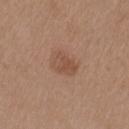{"biopsy_status": "not biopsied; imaged during a skin examination", "site": "right upper arm", "lighting": "white-light", "automated_metrics": {"border_irregularity_0_10": 2.0, "color_variation_0_10": 2.5, "peripheral_color_asymmetry": 1.0}, "patient": {"sex": "female", "age_approx": 40}, "image": {"source": "total-body photography crop", "field_of_view_mm": 15}}